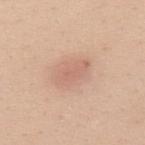Part of a total-body skin-imaging series; this lesion was reviewed on a skin check and was not flagged for biopsy. A female patient, in their mid- to late 20s. A roughly 15 mm field-of-view crop from a total-body skin photograph. Longest diameter approximately 4 mm. Captured under white-light illumination. The lesion is on the chest.